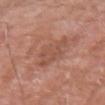Cropped from a total-body skin-imaging series; the visible field is about 15 mm. A male patient, aged approximately 80. Captured under white-light illumination. Approximately 4 mm at its widest. The lesion is on the right upper arm. An algorithmic analysis of the crop reported a border-irregularity index near 5.5/10, a color-variation rating of about 2/10, and radial color variation of about 1.